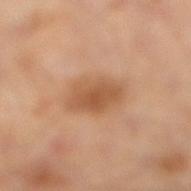Part of a total-body skin-imaging series; this lesion was reviewed on a skin check and was not flagged for biopsy.
Approximately 5 mm at its widest.
The lesion-visualizer software estimated a lesion color around L≈54 a*≈21 b*≈35 in CIELAB. And it measured a border-irregularity rating of about 2/10, a color-variation rating of about 3/10, and a peripheral color-asymmetry measure near 1.
A close-up tile cropped from a whole-body skin photograph, about 15 mm across.
A male patient, aged approximately 50.
This is a cross-polarized tile.
From the left leg.